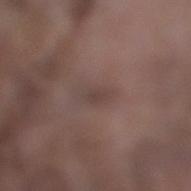acquisition: 15 mm crop, total-body photography | subject: male, in their mid- to late 70s | lighting: white-light illumination | site: the right lower leg | lesion size: ~3 mm (longest diameter).